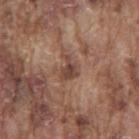Findings:
- biopsy status — imaged on a skin check; not biopsied
- subject — male, roughly 75 years of age
- image source — 15 mm crop, total-body photography
- anatomic site — the mid back